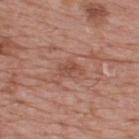Clinical impression:
The lesion was photographed on a routine skin check and not biopsied; there is no pathology result.
Clinical summary:
The lesion is located on the back. Cropped from a whole-body photographic skin survey; the tile spans about 15 mm. A male subject, roughly 75 years of age.